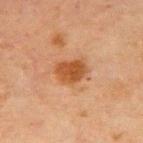| field | value |
|---|---|
| follow-up | no biopsy performed (imaged during a skin exam) |
| acquisition | 15 mm crop, total-body photography |
| body site | the left upper arm |
| lesion diameter | ~3.5 mm (longest diameter) |
| patient | male, roughly 65 years of age |